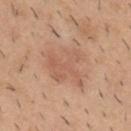No biopsy was performed on this lesion — it was imaged during a full skin examination and was not determined to be concerning.
The subject is a male aged 38–42.
The lesion-visualizer software estimated a classifier nevus-likeness of about 10/100 and a lesion-detection confidence of about 100/100.
Captured under white-light illumination.
Cropped from a whole-body photographic skin survey; the tile spans about 15 mm.
From the mid back.
Measured at roughly 6 mm in maximum diameter.